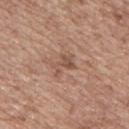{
  "biopsy_status": "not biopsied; imaged during a skin examination",
  "site": "mid back",
  "image": {
    "source": "total-body photography crop",
    "field_of_view_mm": 15
  },
  "lighting": "white-light",
  "patient": {
    "sex": "male",
    "age_approx": 70
  },
  "lesion_size": {
    "long_diameter_mm_approx": 3.0
  }
}